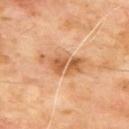No biopsy was performed on this lesion — it was imaged during a full skin examination and was not determined to be concerning. Imaged with cross-polarized lighting. A male patient aged around 65. The lesion is located on the back. An algorithmic analysis of the crop reported a lesion area of about 10 mm², an eccentricity of roughly 0.9, and two-axis asymmetry of about 0.35. The software also gave border irregularity of about 6.5 on a 0–10 scale, internal color variation of about 4.5 on a 0–10 scale, and radial color variation of about 1.5. The analysis additionally found an automated nevus-likeness rating near 0 out of 100 and lesion-presence confidence of about 100/100. A 15 mm close-up extracted from a 3D total-body photography capture.